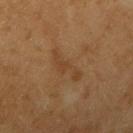<record>
  <biopsy_status>not biopsied; imaged during a skin examination</biopsy_status>
  <patient>
    <sex>male</sex>
    <age_approx>60</age_approx>
  </patient>
  <image>
    <source>total-body photography crop</source>
    <field_of_view_mm>15</field_of_view_mm>
  </image>
  <site>right upper arm</site>
</record>